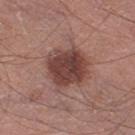Q: Was this lesion biopsied?
A: no biopsy performed (imaged during a skin exam)
Q: Lesion location?
A: the left thigh
Q: What kind of image is this?
A: ~15 mm crop, total-body skin-cancer survey
Q: What lighting was used for the tile?
A: white-light illumination
Q: What did automated image analysis measure?
A: an area of roughly 17 mm², an eccentricity of roughly 0.4, and a symmetry-axis asymmetry near 0.15; about 13 CIELAB-L* units darker than the surrounding skin and a lesion-to-skin contrast of about 10 (normalized; higher = more distinct); a border-irregularity index near 2/10; a classifier nevus-likeness of about 40/100 and a detector confidence of about 100 out of 100 that the crop contains a lesion
Q: What is the lesion's diameter?
A: ≈4.5 mm
Q: Who is the patient?
A: male, roughly 70 years of age